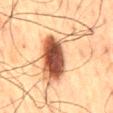Captured during whole-body skin photography for melanoma surveillance; the lesion was not biopsied.
Automated image analysis of the tile measured a footprint of about 26 mm², an outline eccentricity of about 0.9 (0 = round, 1 = elongated), and two-axis asymmetry of about 0.55. It also reported a mean CIELAB color near L≈47 a*≈19 b*≈29 and roughly 17 lightness units darker than nearby skin. The analysis additionally found border irregularity of about 9 on a 0–10 scale and a within-lesion color-variation index near 9.5/10.
A male patient aged around 60.
The tile uses cross-polarized illumination.
Approximately 11 mm at its widest.
The lesion is on the mid back.
A 15 mm close-up extracted from a 3D total-body photography capture.